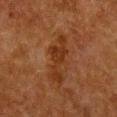{
  "biopsy_status": "not biopsied; imaged during a skin examination",
  "image": {
    "source": "total-body photography crop",
    "field_of_view_mm": 15
  },
  "lighting": "cross-polarized",
  "patient": {
    "sex": "female",
    "age_approx": 50
  },
  "lesion_size": {
    "long_diameter_mm_approx": 6.0
  },
  "site": "chest"
}